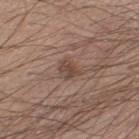Case summary:
• follow-up: imaged on a skin check; not biopsied
• patient: male, aged approximately 40
• body site: the right lower leg
• image source: ~15 mm tile from a whole-body skin photo